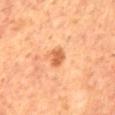Imaged during a routine full-body skin examination; the lesion was not biopsied and no histopathology is available. An algorithmic analysis of the crop reported an area of roughly 4 mm² and an outline eccentricity of about 0.75 (0 = round, 1 = elongated). And it measured a border-irregularity rating of about 2.5/10 and a color-variation rating of about 1.5/10. And it measured a nevus-likeness score of about 75/100 and lesion-presence confidence of about 100/100. The lesion is located on the mid back. Longest diameter approximately 2.5 mm. A 15 mm close-up tile from a total-body photography series done for melanoma screening. A male patient aged 63–67.